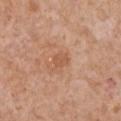Clinical summary:
This image is a 15 mm lesion crop taken from a total-body photograph. The lesion is on the chest. A female subject aged approximately 70.
Diagnosis:
Biopsy histopathology demonstrated a squamous cell carcinoma in situ.